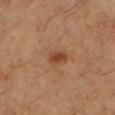A region of skin cropped from a whole-body photographic capture, roughly 15 mm wide.
The subject is a female about 50 years old.
On the left lower leg.
The tile uses cross-polarized illumination.